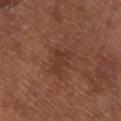notes — imaged on a skin check; not biopsied
acquisition — 15 mm crop, total-body photography
illumination — cross-polarized illumination
size — ≈3 mm
subject — male, about 65 years old
anatomic site — the right lower leg
automated metrics — a footprint of about 5 mm², an eccentricity of roughly 0.75, and a shape-asymmetry score of about 0.35 (0 = symmetric); a mean CIELAB color near L≈35 a*≈22 b*≈26 and about 6 CIELAB-L* units darker than the surrounding skin